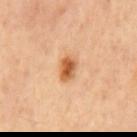Assessment: Captured during whole-body skin photography for melanoma surveillance; the lesion was not biopsied. Background: A region of skin cropped from a whole-body photographic capture, roughly 15 mm wide. The lesion is on the mid back.